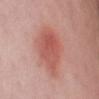Assessment: No biopsy was performed on this lesion — it was imaged during a full skin examination and was not determined to be concerning. Clinical summary: A 15 mm close-up extracted from a 3D total-body photography capture. An algorithmic analysis of the crop reported an outline eccentricity of about 0.8 (0 = round, 1 = elongated) and a symmetry-axis asymmetry near 0.2. A female subject aged around 25. This is a white-light tile. On the chest.